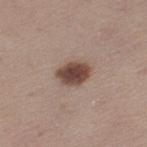{
  "biopsy_status": "not biopsied; imaged during a skin examination",
  "site": "left thigh",
  "lesion_size": {
    "long_diameter_mm_approx": 4.0
  },
  "image": {
    "source": "total-body photography crop",
    "field_of_view_mm": 15
  },
  "lighting": "white-light",
  "patient": {
    "sex": "female",
    "age_approx": 45
  },
  "automated_metrics": {
    "area_mm2_approx": 7.5,
    "nevus_likeness_0_100": 100,
    "lesion_detection_confidence_0_100": 100
  }
}